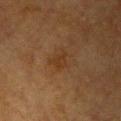Assessment:
Recorded during total-body skin imaging; not selected for excision or biopsy.
Acquisition and patient details:
The subject is a female aged 53–57. On the chest. A roughly 15 mm field-of-view crop from a total-body skin photograph.